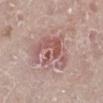Clinical impression:
Recorded during total-body skin imaging; not selected for excision or biopsy.
Acquisition and patient details:
The subject is a female roughly 70 years of age. The lesion-visualizer software estimated a lesion area of about 16 mm², an eccentricity of roughly 0.75, and a shape-asymmetry score of about 0.55 (0 = symmetric). It also reported a lesion color around L≈55 a*≈22 b*≈22 in CIELAB. The software also gave a nevus-likeness score of about 0/100 and lesion-presence confidence of about 100/100. Imaged with white-light lighting. Located on the left lower leg. Approximately 6 mm at its widest. This image is a 15 mm lesion crop taken from a total-body photograph.